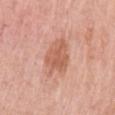  site: left upper arm
  lesion_size:
    long_diameter_mm_approx: 4.0
  image:
    source: total-body photography crop
    field_of_view_mm: 15
  automated_metrics:
    eccentricity: 0.4
    shape_asymmetry: 0.3
    cielab_L: 60
    cielab_a: 25
    cielab_b: 31
    vs_skin_darker_L: 9.0
    color_variation_0_10: 3.0
    peripheral_color_asymmetry: 1.0
  patient:
    sex: female
    age_approx: 65